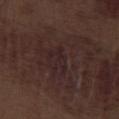workup: no biopsy performed (imaged during a skin exam)
image-analysis metrics: a mean CIELAB color near L≈22 a*≈15 b*≈14 and a lesion-to-skin contrast of about 5.5 (normalized; higher = more distinct); a border-irregularity rating of about 7.5/10 and a within-lesion color-variation index near 0/10; a nevus-likeness score of about 0/100 and a detector confidence of about 50 out of 100 that the crop contains a lesion
patient: male, aged 68 to 72
site: the right lower leg
lesion size: ~3.5 mm (longest diameter)
lighting: white-light
acquisition: total-body-photography crop, ~15 mm field of view